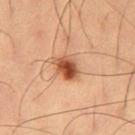biopsy status: catalogued during a skin exam; not biopsied
image: ~15 mm tile from a whole-body skin photo
patient: male, aged 58 to 62
site: the left thigh